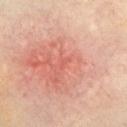Assessment: Imaged during a routine full-body skin examination; the lesion was not biopsied and no histopathology is available.